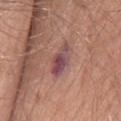Captured during whole-body skin photography for melanoma surveillance; the lesion was not biopsied. The subject is a female aged 63 to 67. A roughly 15 mm field-of-view crop from a total-body skin photograph. Measured at roughly 4.5 mm in maximum diameter. Automated image analysis of the tile measured a shape eccentricity near 0.9 and a shape-asymmetry score of about 0.25 (0 = symmetric). It also reported an average lesion color of about L≈48 a*≈23 b*≈18 (CIELAB), about 10 CIELAB-L* units darker than the surrounding skin, and a normalized lesion–skin contrast near 9.5. The analysis additionally found border irregularity of about 3 on a 0–10 scale and a peripheral color-asymmetry measure near 2. The lesion is located on the back.